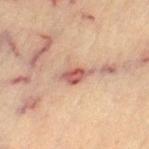Impression: Imaged during a routine full-body skin examination; the lesion was not biopsied and no histopathology is available. Context: An algorithmic analysis of the crop reported an average lesion color of about L≈58 a*≈23 b*≈27 (CIELAB), roughly 12 lightness units darker than nearby skin, and a normalized lesion–skin contrast near 8. And it measured border irregularity of about 3 on a 0–10 scale and internal color variation of about 5 on a 0–10 scale. The patient is a female approximately 65 years of age. The lesion is on the left thigh. Cropped from a total-body skin-imaging series; the visible field is about 15 mm.